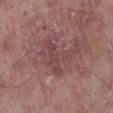Findings:
- notes: catalogued during a skin exam; not biopsied
- image source: ~15 mm crop, total-body skin-cancer survey
- lighting: white-light
- location: the left lower leg
- automated lesion analysis: border irregularity of about 7.5 on a 0–10 scale, a color-variation rating of about 2.5/10, and peripheral color asymmetry of about 1; an automated nevus-likeness rating near 0 out of 100
- subject: female, roughly 75 years of age
- diameter: ~4.5 mm (longest diameter)